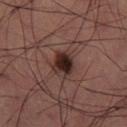Automated tile analysis of the lesion measured a symmetry-axis asymmetry near 0.2. It also reported a lesion color around L≈22 a*≈16 b*≈17 in CIELAB and a normalized lesion–skin contrast near 13. And it measured a border-irregularity index near 2/10, a within-lesion color-variation index near 5/10, and a peripheral color-asymmetry measure near 1.5. A region of skin cropped from a whole-body photographic capture, roughly 15 mm wide. A male patient aged around 60.